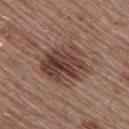workup — no biopsy performed (imaged during a skin exam) | TBP lesion metrics — a lesion area of about 20 mm² and an outline eccentricity of about 0.55 (0 = round, 1 = elongated); roughly 11 lightness units darker than nearby skin and a lesion-to-skin contrast of about 9 (normalized; higher = more distinct); a border-irregularity rating of about 2.5/10 and internal color variation of about 8 on a 0–10 scale | location — the upper back | diameter — ≈5.5 mm | patient — male, aged 53 to 57 | image source — ~15 mm crop, total-body skin-cancer survey | lighting — white-light illumination.